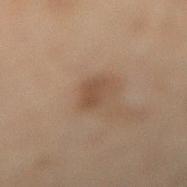follow-up: imaged on a skin check; not biopsied
location: the leg
acquisition: total-body-photography crop, ~15 mm field of view
patient: female, in their mid- to late 50s
size: ≈3 mm
image-analysis metrics: a footprint of about 5 mm², an outline eccentricity of about 0.7 (0 = round, 1 = elongated), and two-axis asymmetry of about 0.25; a lesion color around L≈41 a*≈15 b*≈26 in CIELAB and about 7 CIELAB-L* units darker than the surrounding skin; a border-irregularity index near 2.5/10, internal color variation of about 1.5 on a 0–10 scale, and a peripheral color-asymmetry measure near 0.5